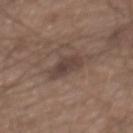Notes:
– biopsy status: no biopsy performed (imaged during a skin exam)
– location: the mid back
– image-analysis metrics: a footprint of about 7 mm², a shape eccentricity near 0.75, and two-axis asymmetry of about 0.25
– image: 15 mm crop, total-body photography
– lesion diameter: ~3.5 mm (longest diameter)
– patient: male, in their mid- to late 60s
– tile lighting: white-light